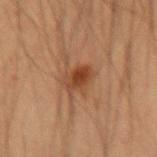{
  "biopsy_status": "not biopsied; imaged during a skin examination",
  "lesion_size": {
    "long_diameter_mm_approx": 3.0
  },
  "site": "right forearm",
  "patient": {
    "sex": "male",
    "age_approx": 35
  },
  "image": {
    "source": "total-body photography crop",
    "field_of_view_mm": 15
  }
}